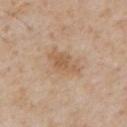Recorded during total-body skin imaging; not selected for excision or biopsy.
Measured at roughly 4.5 mm in maximum diameter.
The lesion is located on the chest.
This image is a 15 mm lesion crop taken from a total-body photograph.
The lesion-visualizer software estimated a lesion area of about 8.5 mm², a shape eccentricity near 0.8, and a shape-asymmetry score of about 0.2 (0 = symmetric). The analysis additionally found an average lesion color of about L≈59 a*≈17 b*≈33 (CIELAB), a lesion–skin lightness drop of about 8, and a normalized border contrast of about 6. And it measured a nevus-likeness score of about 5/100 and lesion-presence confidence of about 100/100.
The patient is a male about 60 years old.